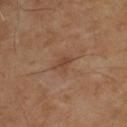{
  "biopsy_status": "not biopsied; imaged during a skin examination",
  "automated_metrics": {
    "lesion_detection_confidence_0_100": 100
  },
  "image": {
    "source": "total-body photography crop",
    "field_of_view_mm": 15
  },
  "lesion_size": {
    "long_diameter_mm_approx": 2.5
  },
  "patient": {
    "sex": "male",
    "age_approx": 55
  },
  "site": "upper back",
  "lighting": "cross-polarized"
}